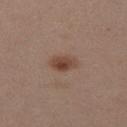Impression:
Imaged during a routine full-body skin examination; the lesion was not biopsied and no histopathology is available.
Acquisition and patient details:
Imaged with white-light lighting. Approximately 3.5 mm at its widest. A 15 mm close-up tile from a total-body photography series done for melanoma screening. Located on the left thigh. Automated image analysis of the tile measured a mean CIELAB color near L≈44 a*≈19 b*≈27 and a normalized lesion–skin contrast near 8.5. The analysis additionally found a border-irregularity rating of about 2.5/10, internal color variation of about 4 on a 0–10 scale, and a peripheral color-asymmetry measure near 1.5. A female patient, aged approximately 30.